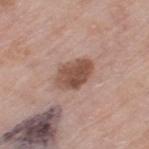Cropped from a total-body skin-imaging series; the visible field is about 15 mm.
The subject is a female aged around 70.
From the left thigh.
On biopsy, histopathology showed a solar lentigo, classified as a benign lesion.